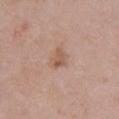Recorded during total-body skin imaging; not selected for excision or biopsy. The lesion's longest dimension is about 2.5 mm. A 15 mm close-up extracted from a 3D total-body photography capture. A female patient, roughly 50 years of age. Imaged with white-light lighting. On the chest. The lesion-visualizer software estimated an area of roughly 3.5 mm² and an outline eccentricity of about 0.75 (0 = round, 1 = elongated).